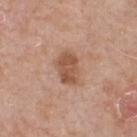The lesion was tiled from a total-body skin photograph and was not biopsied.
Located on the front of the torso.
A male patient, aged around 50.
The lesion-visualizer software estimated an outline eccentricity of about 0.75 (0 = round, 1 = elongated) and a symmetry-axis asymmetry near 0.15. And it measured a mean CIELAB color near L≈53 a*≈21 b*≈31 and a lesion–skin lightness drop of about 11. It also reported a color-variation rating of about 2.5/10.
A 15 mm close-up tile from a total-body photography series done for melanoma screening.
The lesion's longest dimension is about 3.5 mm.
The tile uses white-light illumination.